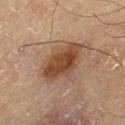Case summary:
• workup · imaged on a skin check; not biopsied
• patient · male, aged approximately 70
• automated metrics · about 10 CIELAB-L* units darker than the surrounding skin and a lesion-to-skin contrast of about 9.5 (normalized; higher = more distinct); border irregularity of about 2.5 on a 0–10 scale and a peripheral color-asymmetry measure near 1; a detector confidence of about 100 out of 100 that the crop contains a lesion
• tile lighting · cross-polarized illumination
• lesion size · ~5.5 mm (longest diameter)
• imaging modality · ~15 mm crop, total-body skin-cancer survey
• location · the leg